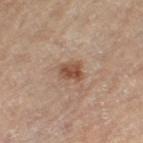Imaged during a routine full-body skin examination; the lesion was not biopsied and no histopathology is available. The tile uses cross-polarized illumination. On the leg. A 15 mm close-up tile from a total-body photography series done for melanoma screening. The subject is a male in their mid-80s or older.